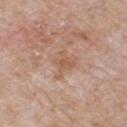Clinical impression: The lesion was photographed on a routine skin check and not biopsied; there is no pathology result. Acquisition and patient details: A roughly 15 mm field-of-view crop from a total-body skin photograph. A male subject, about 60 years old. The lesion is on the front of the torso. This is a white-light tile. The lesion's longest dimension is about 3.5 mm.